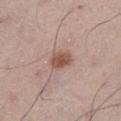Q: Is there a histopathology result?
A: total-body-photography surveillance lesion; no biopsy
Q: What lighting was used for the tile?
A: white-light
Q: How was this image acquired?
A: 15 mm crop, total-body photography
Q: What are the patient's age and sex?
A: male, aged 53–57